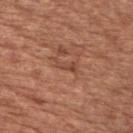Captured during whole-body skin photography for melanoma surveillance; the lesion was not biopsied.
The lesion-visualizer software estimated an area of roughly 3 mm² and an eccentricity of roughly 0.9. The analysis additionally found a nevus-likeness score of about 0/100 and a lesion-detection confidence of about 95/100.
On the chest.
The tile uses white-light illumination.
Cropped from a whole-body photographic skin survey; the tile spans about 15 mm.
A male subject, roughly 65 years of age.